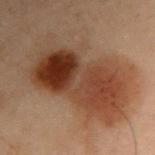Assessment: The lesion was tiled from a total-body skin photograph and was not biopsied. Context: About 11.5 mm across. A male subject, in their mid- to late 50s. This image is a 15 mm lesion crop taken from a total-body photograph. The lesion is on the arm. The lesion-visualizer software estimated about 11 CIELAB-L* units darker than the surrounding skin and a normalized lesion–skin contrast near 10. The analysis additionally found a border-irregularity rating of about 4/10, a within-lesion color-variation index near 9.5/10, and a peripheral color-asymmetry measure near 3.5. The software also gave an automated nevus-likeness rating near 50 out of 100 and lesion-presence confidence of about 100/100. The tile uses cross-polarized illumination.